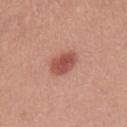workup = total-body-photography surveillance lesion; no biopsy | patient = female, aged 33 to 37 | image source = ~15 mm crop, total-body skin-cancer survey | location = the chest.